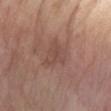Image and clinical context: From the left forearm. Captured under white-light illumination. A female patient, about 75 years old. A close-up tile cropped from a whole-body skin photograph, about 15 mm across. Longest diameter approximately 4 mm.